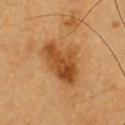follow-up — imaged on a skin check; not biopsied
TBP lesion metrics — a footprint of about 16 mm² and an eccentricity of roughly 0.75; a mean CIELAB color near L≈49 a*≈24 b*≈42, about 12 CIELAB-L* units darker than the surrounding skin, and a normalized border contrast of about 9; a border-irregularity index near 3.5/10, internal color variation of about 6 on a 0–10 scale, and a peripheral color-asymmetry measure near 2.5
acquisition — ~15 mm tile from a whole-body skin photo
lesion diameter — about 6 mm
location — the chest
illumination — cross-polarized illumination
patient — male, aged around 60